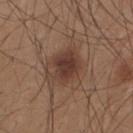A 15 mm close-up tile from a total-body photography series done for melanoma screening. A male patient, in their mid- to late 20s. On the upper back.